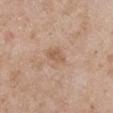| feature | finding |
|---|---|
| notes | catalogued during a skin exam; not biopsied |
| patient | male, aged 48–52 |
| image source | ~15 mm tile from a whole-body skin photo |
| anatomic site | the right upper arm |